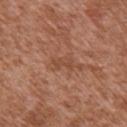biopsy status: no biopsy performed (imaged during a skin exam)
site: the front of the torso
imaging modality: 15 mm crop, total-body photography
automated lesion analysis: an average lesion color of about L≈47 a*≈23 b*≈31 (CIELAB), about 6 CIELAB-L* units darker than the surrounding skin, and a normalized border contrast of about 5; border irregularity of about 4 on a 0–10 scale, internal color variation of about 1 on a 0–10 scale, and peripheral color asymmetry of about 0.5; a nevus-likeness score of about 0/100 and lesion-presence confidence of about 100/100
subject: male, aged around 45
illumination: white-light
size: ~3 mm (longest diameter)